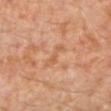The lesion was tiled from a total-body skin photograph and was not biopsied. A 15 mm close-up extracted from a 3D total-body photography capture. An algorithmic analysis of the crop reported a lesion color around L≈57 a*≈23 b*≈35 in CIELAB, roughly 6 lightness units darker than nearby skin, and a normalized lesion–skin contrast near 5. The software also gave a border-irregularity rating of about 5/10 and internal color variation of about 0 on a 0–10 scale. The software also gave a nevus-likeness score of about 0/100 and a lesion-detection confidence of about 100/100. Approximately 3.5 mm at its widest. The subject is a male about 30 years old. The lesion is on the left lower leg. The tile uses cross-polarized illumination.